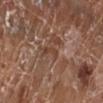workup = imaged on a skin check; not biopsied
lighting = white-light
anatomic site = the leg
size = ~2.5 mm (longest diameter)
acquisition = ~15 mm crop, total-body skin-cancer survey
patient = female, about 75 years old